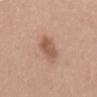This lesion was catalogued during total-body skin photography and was not selected for biopsy. This is a white-light tile. On the mid back. The subject is a male approximately 50 years of age. Approximately 4 mm at its widest. A lesion tile, about 15 mm wide, cut from a 3D total-body photograph. An algorithmic analysis of the crop reported an area of roughly 7 mm² and a shape eccentricity near 0.8. And it measured a classifier nevus-likeness of about 80/100 and a lesion-detection confidence of about 100/100.